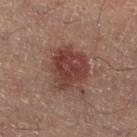Clinical impression:
No biopsy was performed on this lesion — it was imaged during a full skin examination and was not determined to be concerning.
Acquisition and patient details:
Located on the left lower leg. This is a cross-polarized tile. Longest diameter approximately 5.5 mm. A close-up tile cropped from a whole-body skin photograph, about 15 mm across. Automated image analysis of the tile measured a lesion color around L≈35 a*≈20 b*≈21 in CIELAB and a lesion-to-skin contrast of about 8.5 (normalized; higher = more distinct). The software also gave border irregularity of about 2.5 on a 0–10 scale and a peripheral color-asymmetry measure near 1.5. A male subject, approximately 50 years of age.